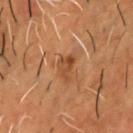Notes:
– follow-up: total-body-photography surveillance lesion; no biopsy
– imaging modality: total-body-photography crop, ~15 mm field of view
– diameter: about 3.5 mm
– body site: the chest
– lighting: cross-polarized illumination
– subject: male, aged approximately 50
– image-analysis metrics: a footprint of about 4.5 mm², an outline eccentricity of about 0.8 (0 = round, 1 = elongated), and a shape-asymmetry score of about 0.4 (0 = symmetric); an average lesion color of about L≈37 a*≈20 b*≈30 (CIELAB) and a normalized lesion–skin contrast near 6.5; a border-irregularity index near 4/10, internal color variation of about 4 on a 0–10 scale, and peripheral color asymmetry of about 1.5; a classifier nevus-likeness of about 0/100 and a detector confidence of about 100 out of 100 that the crop contains a lesion